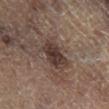The recorded lesion diameter is about 4 mm.
A male subject, aged 63–67.
Cropped from a total-body skin-imaging series; the visible field is about 15 mm.
On the right lower leg.
This is a cross-polarized tile.
An algorithmic analysis of the crop reported a lesion area of about 10 mm², an outline eccentricity of about 0.75 (0 = round, 1 = elongated), and a shape-asymmetry score of about 0.25 (0 = symmetric). And it measured an average lesion color of about L≈34 a*≈14 b*≈19 (CIELAB) and about 10 CIELAB-L* units darker than the surrounding skin. The analysis additionally found border irregularity of about 3 on a 0–10 scale and a color-variation rating of about 4/10. And it measured a classifier nevus-likeness of about 20/100 and lesion-presence confidence of about 100/100.